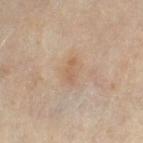<record>
<biopsy_status>not biopsied; imaged during a skin examination</biopsy_status>
<site>leg</site>
<lighting>cross-polarized</lighting>
<lesion_size>
  <long_diameter_mm_approx>3.0</long_diameter_mm_approx>
</lesion_size>
<image>
  <source>total-body photography crop</source>
  <field_of_view_mm>15</field_of_view_mm>
</image>
<patient>
  <sex>female</sex>
  <age_approx>50</age_approx>
</patient>
</record>